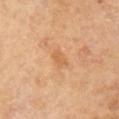| field | value |
|---|---|
| biopsy status | imaged on a skin check; not biopsied |
| imaging modality | 15 mm crop, total-body photography |
| subject | male, aged 68–72 |
| illumination | cross-polarized |
| diameter | about 2.5 mm |
| body site | the arm |